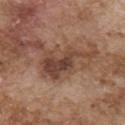biopsy status=no biopsy performed (imaged during a skin exam)
patient=male, in their mid- to late 70s
location=the chest
tile lighting=white-light
imaging modality=total-body-photography crop, ~15 mm field of view
size=~7.5 mm (longest diameter)